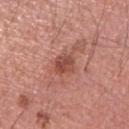  image:
    source: total-body photography crop
    field_of_view_mm: 15
  automated_metrics:
    area_mm2_approx: 4.5
    eccentricity: 0.55
    shape_asymmetry: 0.2
    nevus_likeness_0_100: 25
    lesion_detection_confidence_0_100: 100
  lesion_size:
    long_diameter_mm_approx: 2.5
  patient:
    sex: male
    age_approx: 40
  site: left upper arm